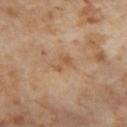follow-up: total-body-photography surveillance lesion; no biopsy | automated lesion analysis: an area of roughly 3 mm², an outline eccentricity of about 0.8 (0 = round, 1 = elongated), and a shape-asymmetry score of about 0.6 (0 = symmetric); roughly 7 lightness units darker than nearby skin and a lesion-to-skin contrast of about 6 (normalized; higher = more distinct); lesion-presence confidence of about 100/100 | lighting: cross-polarized illumination | patient: female, approximately 55 years of age | image source: ~15 mm tile from a whole-body skin photo | lesion size: about 2.5 mm | location: the left thigh.